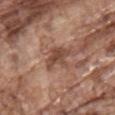This lesion was catalogued during total-body skin photography and was not selected for biopsy.